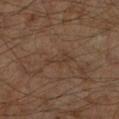Recorded during total-body skin imaging; not selected for excision or biopsy.